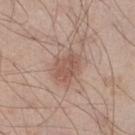Impression: No biopsy was performed on this lesion — it was imaged during a full skin examination and was not determined to be concerning. Acquisition and patient details: A 15 mm crop from a total-body photograph taken for skin-cancer surveillance. The lesion-visualizer software estimated a mean CIELAB color near L≈54 a*≈20 b*≈26, about 9 CIELAB-L* units darker than the surrounding skin, and a normalized lesion–skin contrast near 6. The software also gave border irregularity of about 2.5 on a 0–10 scale, internal color variation of about 2.5 on a 0–10 scale, and peripheral color asymmetry of about 1. The tile uses white-light illumination. The patient is a male roughly 35 years of age. The lesion is on the right lower leg. Longest diameter approximately 3.5 mm.